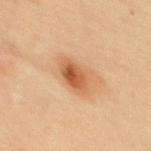- workup: no biopsy performed (imaged during a skin exam)
- acquisition: ~15 mm crop, total-body skin-cancer survey
- site: the upper back
- subject: female, in their mid-30s